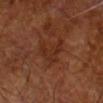Notes:
– notes: imaged on a skin check; not biopsied
– patient: male, in their mid- to late 60s
– lesion size: about 5 mm
– lighting: cross-polarized
– imaging modality: total-body-photography crop, ~15 mm field of view
– image-analysis metrics: a mean CIELAB color near L≈29 a*≈23 b*≈29, about 6 CIELAB-L* units darker than the surrounding skin, and a normalized border contrast of about 6; a nevus-likeness score of about 0/100 and a detector confidence of about 90 out of 100 that the crop contains a lesion